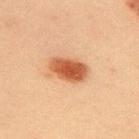This lesion was catalogued during total-body skin photography and was not selected for biopsy. The lesion-visualizer software estimated an average lesion color of about L≈47 a*≈24 b*≈34 (CIELAB) and a lesion–skin lightness drop of about 14. Imaged with cross-polarized lighting. From the upper back. A roughly 15 mm field-of-view crop from a total-body skin photograph. Measured at roughly 4.5 mm in maximum diameter. A male subject aged 58 to 62.